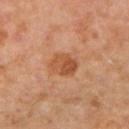Clinical impression: Captured during whole-body skin photography for melanoma surveillance; the lesion was not biopsied. Clinical summary: The subject is a female roughly 50 years of age. On the left upper arm. A 15 mm close-up tile from a total-body photography series done for melanoma screening.